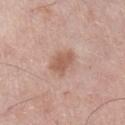image:
  source: total-body photography crop
  field_of_view_mm: 15
lesion_size:
  long_diameter_mm_approx: 3.5
site: left lower leg
patient:
  sex: male
  age_approx: 70
automated_metrics:
  border_irregularity_0_10: 2.0
  color_variation_0_10: 1.5
  nevus_likeness_0_100: 15
  lesion_detection_confidence_0_100: 100
lighting: white-light